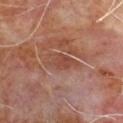{"biopsy_status": "not biopsied; imaged during a skin examination", "site": "chest", "lesion_size": {"long_diameter_mm_approx": 4.5}, "lighting": "cross-polarized", "automated_metrics": {"border_irregularity_0_10": 3.0, "color_variation_0_10": 3.0, "peripheral_color_asymmetry": 0.5, "nevus_likeness_0_100": 5}, "image": {"source": "total-body photography crop", "field_of_view_mm": 15}, "patient": {"sex": "male", "age_approx": 65}}